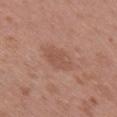No biopsy was performed on this lesion — it was imaged during a full skin examination and was not determined to be concerning.
A region of skin cropped from a whole-body photographic capture, roughly 15 mm wide.
On the right upper arm.
A male patient, about 50 years old.